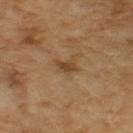Case summary:
• notes: no biopsy performed (imaged during a skin exam)
• tile lighting: cross-polarized
• image: ~15 mm tile from a whole-body skin photo
• patient: male, aged approximately 65
• image-analysis metrics: a lesion color around L≈43 a*≈17 b*≈33 in CIELAB and a lesion-to-skin contrast of about 7 (normalized; higher = more distinct); a classifier nevus-likeness of about 30/100